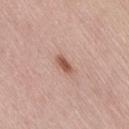Q: Is there a histopathology result?
A: no biopsy performed (imaged during a skin exam)
Q: How was this image acquired?
A: ~15 mm tile from a whole-body skin photo
Q: What lighting was used for the tile?
A: white-light illumination
Q: Who is the patient?
A: female, approximately 65 years of age
Q: What is the lesion's diameter?
A: about 2.5 mm
Q: Where on the body is the lesion?
A: the right thigh